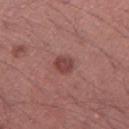workup: catalogued during a skin exam; not biopsied
patient: male, in their mid-40s
diameter: about 2.5 mm
image: 15 mm crop, total-body photography
tile lighting: white-light illumination
image-analysis metrics: a lesion area of about 5 mm², an outline eccentricity of about 0.55 (0 = round, 1 = elongated), and a symmetry-axis asymmetry near 0.15; a lesion color around L≈44 a*≈25 b*≈22 in CIELAB, a lesion–skin lightness drop of about 10, and a normalized border contrast of about 7.5; border irregularity of about 1.5 on a 0–10 scale; a classifier nevus-likeness of about 85/100 and a lesion-detection confidence of about 100/100
anatomic site: the right thigh